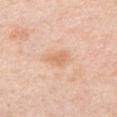patient: male, about 50 years old; acquisition: total-body-photography crop, ~15 mm field of view; anatomic site: the chest.